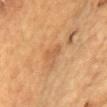The lesion was tiled from a total-body skin photograph and was not biopsied. The lesion is located on the chest. Captured under cross-polarized illumination. The lesion-visualizer software estimated a lesion area of about 3 mm², a shape eccentricity near 0.85, and two-axis asymmetry of about 0.4. And it measured border irregularity of about 4 on a 0–10 scale, internal color variation of about 0.5 on a 0–10 scale, and peripheral color asymmetry of about 0. The software also gave an automated nevus-likeness rating near 0 out of 100 and lesion-presence confidence of about 100/100. A roughly 15 mm field-of-view crop from a total-body skin photograph. The recorded lesion diameter is about 2.5 mm. A male subject roughly 85 years of age or older.